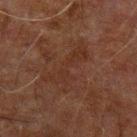Q: How was this image acquired?
A: ~15 mm tile from a whole-body skin photo
Q: What are the patient's age and sex?
A: male, in their 60s
Q: Lesion size?
A: ≈6 mm
Q: What is the anatomic site?
A: the left upper arm
Q: How was the tile lit?
A: cross-polarized illumination